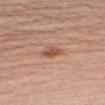Findings:
• biopsy status · imaged on a skin check; not biopsied
• lesion size · ~3 mm (longest diameter)
• lighting · white-light
• image source · ~15 mm crop, total-body skin-cancer survey
• automated metrics · an area of roughly 4 mm², an outline eccentricity of about 0.85 (0 = round, 1 = elongated), and two-axis asymmetry of about 0.25; a lesion color around L≈53 a*≈23 b*≈31 in CIELAB and about 11 CIELAB-L* units darker than the surrounding skin; a border-irregularity index near 3/10 and peripheral color asymmetry of about 1.5; a lesion-detection confidence of about 100/100
• anatomic site · the left upper arm
• subject · male, roughly 60 years of age